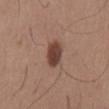Impression: The lesion was tiled from a total-body skin photograph and was not biopsied. Context: A roughly 15 mm field-of-view crop from a total-body skin photograph. This is a white-light tile. The patient is a male roughly 65 years of age. About 4 mm across. From the mid back.